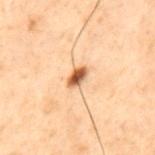Automated image analysis of the tile measured an area of roughly 3.5 mm², an eccentricity of roughly 0.85, and two-axis asymmetry of about 0.2. The software also gave a lesion color around L≈46 a*≈19 b*≈31 in CIELAB, roughly 16 lightness units darker than nearby skin, and a normalized border contrast of about 11.5. And it measured an automated nevus-likeness rating near 100 out of 100 and lesion-presence confidence of about 100/100. The patient is a male aged 68–72. This is a cross-polarized tile. A 15 mm crop from a total-body photograph taken for skin-cancer surveillance. From the upper back.